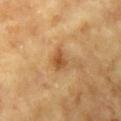This lesion was catalogued during total-body skin photography and was not selected for biopsy.
The lesion's longest dimension is about 2.5 mm.
Cropped from a total-body skin-imaging series; the visible field is about 15 mm.
The subject is a female aged around 75.
Imaged with cross-polarized lighting.
Automated tile analysis of the lesion measured a classifier nevus-likeness of about 15/100 and lesion-presence confidence of about 100/100.
The lesion is located on the right upper arm.